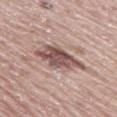Impression:
Captured during whole-body skin photography for melanoma surveillance; the lesion was not biopsied.
Image and clinical context:
Automated image analysis of the tile measured border irregularity of about 4 on a 0–10 scale, internal color variation of about 6 on a 0–10 scale, and a peripheral color-asymmetry measure near 2. This image is a 15 mm lesion crop taken from a total-body photograph. A male patient about 65 years old. From the left thigh. The recorded lesion diameter is about 6 mm. Imaged with white-light lighting.